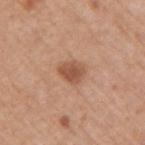| field | value |
|---|---|
| follow-up | catalogued during a skin exam; not biopsied |
| site | the right upper arm |
| patient | male, in their mid-60s |
| tile lighting | white-light |
| image | 15 mm crop, total-body photography |